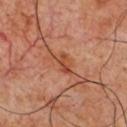Captured during whole-body skin photography for melanoma surveillance; the lesion was not biopsied. Measured at roughly 3 mm in maximum diameter. A male subject aged 58–62. The lesion-visualizer software estimated roughly 8 lightness units darker than nearby skin and a lesion-to-skin contrast of about 6.5 (normalized; higher = more distinct). The analysis additionally found a border-irregularity index near 5/10, a color-variation rating of about 4/10, and radial color variation of about 1.5. It also reported a nevus-likeness score of about 0/100 and a detector confidence of about 100 out of 100 that the crop contains a lesion. A roughly 15 mm field-of-view crop from a total-body skin photograph. The lesion is on the chest.